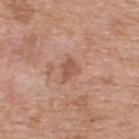workup: total-body-photography surveillance lesion; no biopsy
anatomic site: the upper back
acquisition: total-body-photography crop, ~15 mm field of view
subject: male, in their mid- to late 50s